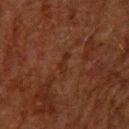No biopsy was performed on this lesion — it was imaged during a full skin examination and was not determined to be concerning. The lesion is located on the upper back. A 15 mm close-up tile from a total-body photography series done for melanoma screening. The lesion's longest dimension is about 3 mm. A male subject, in their 60s.